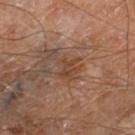workup = catalogued during a skin exam; not biopsied.